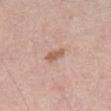Imaged during a routine full-body skin examination; the lesion was not biopsied and no histopathology is available.
The lesion's longest dimension is about 2.5 mm.
This image is a 15 mm lesion crop taken from a total-body photograph.
The patient is a male aged around 60.
From the front of the torso.
This is a white-light tile.